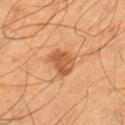Captured during whole-body skin photography for melanoma surveillance; the lesion was not biopsied. Captured under cross-polarized illumination. A 15 mm crop from a total-body photograph taken for skin-cancer surveillance. The recorded lesion diameter is about 4 mm. The lesion-visualizer software estimated an area of roughly 7.5 mm² and a shape eccentricity near 0.8. And it measured an average lesion color of about L≈54 a*≈25 b*≈39 (CIELAB) and a lesion–skin lightness drop of about 12. The software also gave a border-irregularity index near 3/10, internal color variation of about 3 on a 0–10 scale, and a peripheral color-asymmetry measure near 1. The analysis additionally found an automated nevus-likeness rating near 65 out of 100 and lesion-presence confidence of about 100/100. A male patient roughly 55 years of age. The lesion is on the left thigh.